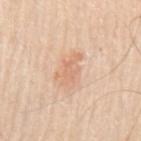Impression: The lesion was tiled from a total-body skin photograph and was not biopsied. Acquisition and patient details: Located on the left upper arm. The lesion's longest dimension is about 4 mm. A 15 mm close-up extracted from a 3D total-body photography capture. A male subject about 70 years old. Imaged with white-light lighting. Automated image analysis of the tile measured a footprint of about 6.5 mm² and an eccentricity of roughly 0.8. The analysis additionally found an average lesion color of about L≈69 a*≈21 b*≈33 (CIELAB), about 8 CIELAB-L* units darker than the surrounding skin, and a lesion-to-skin contrast of about 5 (normalized; higher = more distinct). The analysis additionally found border irregularity of about 5.5 on a 0–10 scale, a within-lesion color-variation index near 2/10, and a peripheral color-asymmetry measure near 0.5.